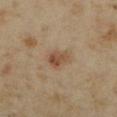notes: catalogued during a skin exam; not biopsied | lesion diameter: ≈3 mm | image source: ~15 mm crop, total-body skin-cancer survey | anatomic site: the right upper arm | tile lighting: cross-polarized illumination | patient: female, approximately 35 years of age.